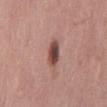This lesion was catalogued during total-body skin photography and was not selected for biopsy. On the mid back. A male patient aged 58 to 62. A region of skin cropped from a whole-body photographic capture, roughly 15 mm wide.